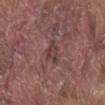  biopsy_status: not biopsied; imaged during a skin examination
  image:
    source: total-body photography crop
    field_of_view_mm: 15
  patient:
    sex: male
    age_approx: 80
  site: back
  lighting: white-light